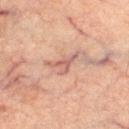Recorded during total-body skin imaging; not selected for excision or biopsy.
Located on the left thigh.
Cropped from a whole-body photographic skin survey; the tile spans about 15 mm.
A male patient, aged approximately 60.